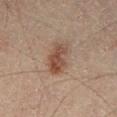Case summary:
- follow-up: imaged on a skin check; not biopsied
- patient: male, aged 38 to 42
- anatomic site: the left thigh
- tile lighting: cross-polarized illumination
- imaging modality: 15 mm crop, total-body photography